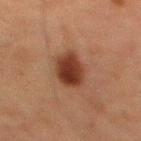biopsy status = total-body-photography surveillance lesion; no biopsy | imaging modality = ~15 mm crop, total-body skin-cancer survey | tile lighting = cross-polarized | lesion size = ≈5 mm | patient = male, aged 58 to 62 | site = the upper back.